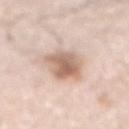  biopsy_status: not biopsied; imaged during a skin examination